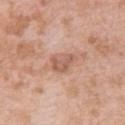notes=no biopsy performed (imaged during a skin exam) | location=the back | image=~15 mm tile from a whole-body skin photo | subject=female, about 40 years old.